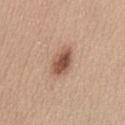<record>
<biopsy_status>not biopsied; imaged during a skin examination</biopsy_status>
<site>abdomen</site>
<patient>
  <sex>female</sex>
  <age_approx>35</age_approx>
</patient>
<image>
  <source>total-body photography crop</source>
  <field_of_view_mm>15</field_of_view_mm>
</image>
</record>